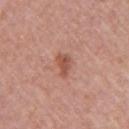Recorded during total-body skin imaging; not selected for excision or biopsy.
The lesion is located on the right upper arm.
An algorithmic analysis of the crop reported an area of roughly 4 mm², an outline eccentricity of about 0.6 (0 = round, 1 = elongated), and two-axis asymmetry of about 0.4. The analysis additionally found a lesion color around L≈53 a*≈25 b*≈29 in CIELAB. And it measured a classifier nevus-likeness of about 65/100 and a lesion-detection confidence of about 100/100.
A 15 mm close-up tile from a total-body photography series done for melanoma screening.
A female patient, aged 58–62.